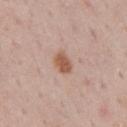This lesion was catalogued during total-body skin photography and was not selected for biopsy. The lesion is on the mid back. A 15 mm close-up extracted from a 3D total-body photography capture. The recorded lesion diameter is about 3 mm. Imaged with white-light lighting. The total-body-photography lesion software estimated an area of roughly 4.5 mm², an outline eccentricity of about 0.75 (0 = round, 1 = elongated), and a symmetry-axis asymmetry near 0.2. The software also gave a lesion–skin lightness drop of about 11 and a lesion-to-skin contrast of about 8.5 (normalized; higher = more distinct). It also reported a border-irregularity index near 1.5/10, a color-variation rating of about 2.5/10, and a peripheral color-asymmetry measure near 1. It also reported a nevus-likeness score of about 95/100 and a lesion-detection confidence of about 100/100. The patient is a male aged around 50.